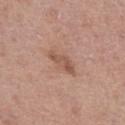workup: no biopsy performed (imaged during a skin exam) | imaging modality: ~15 mm crop, total-body skin-cancer survey | image-analysis metrics: an average lesion color of about L≈53 a*≈21 b*≈28 (CIELAB), about 9 CIELAB-L* units darker than the surrounding skin, and a normalized border contrast of about 6.5; border irregularity of about 4 on a 0–10 scale, a color-variation rating of about 2.5/10, and a peripheral color-asymmetry measure near 0.5; a nevus-likeness score of about 10/100 | anatomic site: the left thigh | lesion size: about 4 mm | tile lighting: white-light | patient: female, aged around 40.